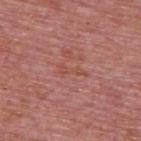{
  "biopsy_status": "not biopsied; imaged during a skin examination",
  "site": "upper back",
  "patient": {
    "sex": "male",
    "age_approx": 75
  },
  "lesion_size": {
    "long_diameter_mm_approx": 3.5
  },
  "lighting": "white-light",
  "image": {
    "source": "total-body photography crop",
    "field_of_view_mm": 15
  }
}